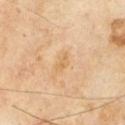No biopsy was performed on this lesion — it was imaged during a full skin examination and was not determined to be concerning. A male patient in their 70s. The recorded lesion diameter is about 2.5 mm. The lesion is on the chest. The lesion-visualizer software estimated a lesion area of about 2.5 mm², an outline eccentricity of about 0.9 (0 = round, 1 = elongated), and two-axis asymmetry of about 0.25. And it measured a lesion color around L≈66 a*≈18 b*≈41 in CIELAB, about 6 CIELAB-L* units darker than the surrounding skin, and a normalized lesion–skin contrast near 5.5. The software also gave a border-irregularity index near 2.5/10, a color-variation rating of about 0/10, and a peripheral color-asymmetry measure near 0. And it measured an automated nevus-likeness rating near 0 out of 100. A close-up tile cropped from a whole-body skin photograph, about 15 mm across. The tile uses cross-polarized illumination.